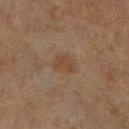Captured during whole-body skin photography for melanoma surveillance; the lesion was not biopsied. The lesion's longest dimension is about 3 mm. A roughly 15 mm field-of-view crop from a total-body skin photograph. On the right lower leg. The patient is a female aged approximately 55.